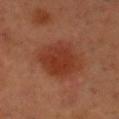biopsy status=catalogued during a skin exam; not biopsied
anatomic site=the head or neck
patient=male, aged around 55
acquisition=~15 mm tile from a whole-body skin photo